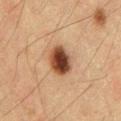Notes:
– biopsy status: total-body-photography surveillance lesion; no biopsy
– subject: male, aged around 60
– tile lighting: cross-polarized illumination
– image-analysis metrics: a nevus-likeness score of about 100/100 and lesion-presence confidence of about 100/100
– image: total-body-photography crop, ~15 mm field of view